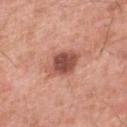follow-up = catalogued during a skin exam; not biopsied
image = 15 mm crop, total-body photography
anatomic site = the right lower leg
subject = male, approximately 80 years of age